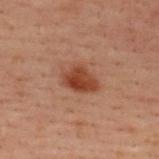Clinical summary:
A 15 mm close-up tile from a total-body photography series done for melanoma screening. The lesion-visualizer software estimated an area of roughly 8.5 mm², an outline eccentricity of about 0.75 (0 = round, 1 = elongated), and two-axis asymmetry of about 0.2. The analysis additionally found a mean CIELAB color near L≈35 a*≈21 b*≈27, a lesion–skin lightness drop of about 9, and a normalized border contrast of about 9. The software also gave a classifier nevus-likeness of about 100/100 and a detector confidence of about 100 out of 100 that the crop contains a lesion. The subject is a male roughly 40 years of age. Located on the upper back.